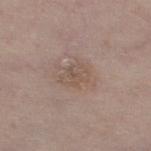workup: imaged on a skin check; not biopsied.